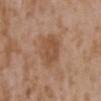Clinical impression:
Part of a total-body skin-imaging series; this lesion was reviewed on a skin check and was not flagged for biopsy.
Context:
From the back. A female patient approximately 35 years of age. The tile uses white-light illumination. About 4.5 mm across. Cropped from a total-body skin-imaging series; the visible field is about 15 mm.